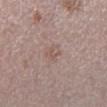Assessment:
Part of a total-body skin-imaging series; this lesion was reviewed on a skin check and was not flagged for biopsy.
Context:
An algorithmic analysis of the crop reported a border-irregularity rating of about 3/10, a within-lesion color-variation index near 3/10, and radial color variation of about 1. And it measured an automated nevus-likeness rating near 0 out of 100 and lesion-presence confidence of about 100/100. On the right lower leg. A female patient about 65 years old. About 2.5 mm across. This image is a 15 mm lesion crop taken from a total-body photograph. The tile uses white-light illumination.